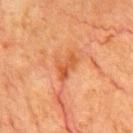The lesion was tiled from a total-body skin photograph and was not biopsied.
Cropped from a total-body skin-imaging series; the visible field is about 15 mm.
Captured under cross-polarized illumination.
Approximately 3.5 mm at its widest.
A male subject aged 78–82.
Automated tile analysis of the lesion measured a classifier nevus-likeness of about 0/100 and a lesion-detection confidence of about 100/100.
Located on the chest.